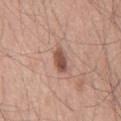{"biopsy_status": "not biopsied; imaged during a skin examination", "site": "chest", "patient": {"sex": "male", "age_approx": 55}, "image": {"source": "total-body photography crop", "field_of_view_mm": 15}}